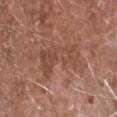workup = catalogued during a skin exam; not biopsied.